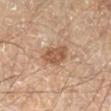{
  "biopsy_status": "not biopsied; imaged during a skin examination",
  "lesion_size": {
    "long_diameter_mm_approx": 4.0
  },
  "image": {
    "source": "total-body photography crop",
    "field_of_view_mm": 15
  },
  "site": "left lower leg",
  "patient": {
    "sex": "male",
    "age_approx": 45
  }
}